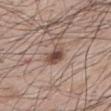No biopsy was performed on this lesion — it was imaged during a full skin examination and was not determined to be concerning.
The recorded lesion diameter is about 3.5 mm.
Cropped from a whole-body photographic skin survey; the tile spans about 15 mm.
The lesion is located on the upper back.
The patient is a male about 65 years old.
An algorithmic analysis of the crop reported an eccentricity of roughly 0.9 and two-axis asymmetry of about 0.35. It also reported a mean CIELAB color near L≈48 a*≈18 b*≈25, about 13 CIELAB-L* units darker than the surrounding skin, and a lesion-to-skin contrast of about 9 (normalized; higher = more distinct).
The tile uses white-light illumination.